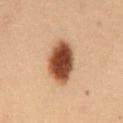Part of a total-body skin-imaging series; this lesion was reviewed on a skin check and was not flagged for biopsy.
The recorded lesion diameter is about 5 mm.
Located on the abdomen.
A 15 mm crop from a total-body photograph taken for skin-cancer surveillance.
The subject is a male in their mid-50s.